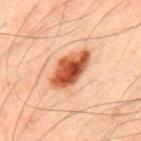Clinical impression:
No biopsy was performed on this lesion — it was imaged during a full skin examination and was not determined to be concerning.
Background:
This is a cross-polarized tile. Automated image analysis of the tile measured a lesion area of about 12 mm² and two-axis asymmetry of about 0.2. And it measured a lesion color around L≈56 a*≈31 b*≈40 in CIELAB, a lesion–skin lightness drop of about 20, and a normalized lesion–skin contrast near 12.5. It also reported a border-irregularity rating of about 2/10, a color-variation rating of about 8.5/10, and peripheral color asymmetry of about 3. And it measured a detector confidence of about 100 out of 100 that the crop contains a lesion. Longest diameter approximately 5.5 mm. A male subject approximately 50 years of age. The lesion is on the upper back. A region of skin cropped from a whole-body photographic capture, roughly 15 mm wide.